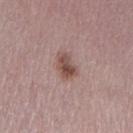Impression: The lesion was photographed on a routine skin check and not biopsied; there is no pathology result. Context: The lesion is located on the left lower leg. A region of skin cropped from a whole-body photographic capture, roughly 15 mm wide. The patient is a female about 50 years old.